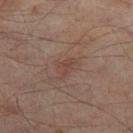Assessment: The lesion was tiled from a total-body skin photograph and was not biopsied. Background: Automated tile analysis of the lesion measured a footprint of about 3.5 mm² and a symmetry-axis asymmetry near 0.4. The analysis additionally found an average lesion color of about L≈41 a*≈18 b*≈23 (CIELAB), about 5 CIELAB-L* units darker than the surrounding skin, and a normalized border contrast of about 5. The software also gave a classifier nevus-likeness of about 5/100. On the right thigh. The patient is a male aged 63 to 67. Captured under cross-polarized illumination. A region of skin cropped from a whole-body photographic capture, roughly 15 mm wide. The lesion's longest dimension is about 2.5 mm.